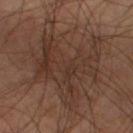  biopsy_status: not biopsied; imaged during a skin examination
  lesion_size:
    long_diameter_mm_approx: 10.0
  image:
    source: total-body photography crop
    field_of_view_mm: 15
  lighting: cross-polarized
  automated_metrics:
    border_irregularity_0_10: 8.0
    color_variation_0_10: 3.5
    peripheral_color_asymmetry: 1.0
    lesion_detection_confidence_0_100: 55
  patient:
    sex: male
    age_approx: 55
  site: left thigh